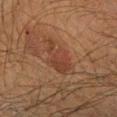Impression: Captured during whole-body skin photography for melanoma surveillance; the lesion was not biopsied. Acquisition and patient details: Approximately 4.5 mm at its widest. An algorithmic analysis of the crop reported an average lesion color of about L≈32 a*≈19 b*≈25 (CIELAB), about 6 CIELAB-L* units darker than the surrounding skin, and a normalized border contrast of about 6. And it measured a border-irregularity rating of about 4/10, internal color variation of about 2.5 on a 0–10 scale, and radial color variation of about 0.5. And it measured a detector confidence of about 100 out of 100 that the crop contains a lesion. A roughly 15 mm field-of-view crop from a total-body skin photograph. A male patient aged around 50. This is a cross-polarized tile. From the left forearm.